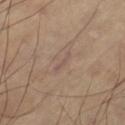Case summary:
– follow-up: catalogued during a skin exam; not biopsied
– acquisition: 15 mm crop, total-body photography
– body site: the leg
– diameter: about 3 mm
– lighting: cross-polarized